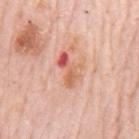Imaged during a routine full-body skin examination; the lesion was not biopsied and no histopathology is available. Approximately 4.5 mm at its widest. From the mid back. A male subject, aged approximately 80. A roughly 15 mm field-of-view crop from a total-body skin photograph. The total-body-photography lesion software estimated an average lesion color of about L≈63 a*≈28 b*≈32 (CIELAB), roughly 11 lightness units darker than nearby skin, and a normalized border contrast of about 7. And it measured a detector confidence of about 100 out of 100 that the crop contains a lesion.